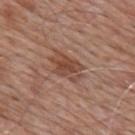notes: total-body-photography surveillance lesion; no biopsy | patient: male, roughly 80 years of age | anatomic site: the upper back | TBP lesion metrics: a footprint of about 9 mm², a shape eccentricity near 0.8, and a shape-asymmetry score of about 0.25 (0 = symmetric); an average lesion color of about L≈46 a*≈21 b*≈28 (CIELAB), roughly 9 lightness units darker than nearby skin, and a lesion-to-skin contrast of about 7 (normalized; higher = more distinct); a lesion-detection confidence of about 100/100 | illumination: white-light | image: ~15 mm crop, total-body skin-cancer survey | lesion diameter: about 4.5 mm.